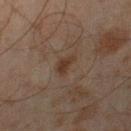Recorded during total-body skin imaging; not selected for excision or biopsy. This is a cross-polarized tile. The lesion is located on the right thigh. Approximately 2.5 mm at its widest. A male subject about 45 years old. A 15 mm crop from a total-body photograph taken for skin-cancer surveillance.